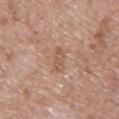This lesion was catalogued during total-body skin photography and was not selected for biopsy. Captured under white-light illumination. A male patient, roughly 50 years of age. The lesion is located on the chest. A roughly 15 mm field-of-view crop from a total-body skin photograph.